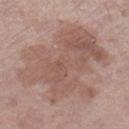Imaged during a routine full-body skin examination; the lesion was not biopsied and no histopathology is available. Cropped from a whole-body photographic skin survey; the tile spans about 15 mm. The lesion is on the right lower leg. Measured at roughly 10.5 mm in maximum diameter. The lesion-visualizer software estimated a lesion color around L≈55 a*≈18 b*≈24 in CIELAB, a lesion–skin lightness drop of about 8, and a normalized border contrast of about 6. The analysis additionally found border irregularity of about 6.5 on a 0–10 scale and a peripheral color-asymmetry measure near 1.5. The patient is a female in their mid- to late 60s.